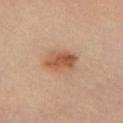<lesion>
<biopsy_status>not biopsied; imaged during a skin examination</biopsy_status>
<lesion_size>
  <long_diameter_mm_approx>4.5</long_diameter_mm_approx>
</lesion_size>
<lighting>cross-polarized</lighting>
<automated_metrics>
  <cielab_L>55</cielab_L>
  <cielab_a>22</cielab_a>
  <cielab_b>34</cielab_b>
  <vs_skin_contrast_norm>8.0</vs_skin_contrast_norm>
</automated_metrics>
<site>left lower leg</site>
<patient>
  <sex>male</sex>
  <age_approx>40</age_approx>
</patient>
<image>
  <source>total-body photography crop</source>
  <field_of_view_mm>15</field_of_view_mm>
</image>
</lesion>